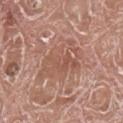Assessment: Recorded during total-body skin imaging; not selected for excision or biopsy. Context: The lesion is on the right lower leg. A 15 mm close-up tile from a total-body photography series done for melanoma screening. The tile uses white-light illumination. An algorithmic analysis of the crop reported an average lesion color of about L≈54 a*≈22 b*≈28 (CIELAB), roughly 7 lightness units darker than nearby skin, and a normalized lesion–skin contrast near 5. The software also gave a lesion-detection confidence of about 90/100. A male subject aged 73 to 77.